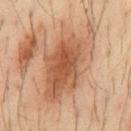The lesion was photographed on a routine skin check and not biopsied; there is no pathology result. A region of skin cropped from a whole-body photographic capture, roughly 15 mm wide. The subject is a male roughly 35 years of age. Captured under cross-polarized illumination. The total-body-photography lesion software estimated a border-irregularity index near 5/10 and peripheral color asymmetry of about 1.5. And it measured a nevus-likeness score of about 100/100. The lesion is on the front of the torso. Approximately 10 mm at its widest.